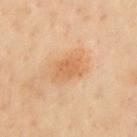Imaged during a routine full-body skin examination; the lesion was not biopsied and no histopathology is available. Longest diameter approximately 4 mm. This is a cross-polarized tile. The subject is a female aged approximately 60. A roughly 15 mm field-of-view crop from a total-body skin photograph. The lesion is located on the front of the torso.